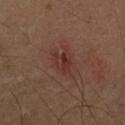biopsy status = no biopsy performed (imaged during a skin exam) | illumination = cross-polarized illumination | patient = male, aged 53 to 57 | site = the arm | size = ≈3 mm | TBP lesion metrics = a lesion color around L≈31 a*≈21 b*≈21 in CIELAB | acquisition = 15 mm crop, total-body photography.